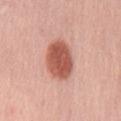{
  "patient": {
    "sex": "female",
    "age_approx": 55
  },
  "site": "mid back",
  "image": {
    "source": "total-body photography crop",
    "field_of_view_mm": 15
  }
}